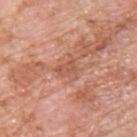– follow-up — catalogued during a skin exam; not biopsied
– location — the upper back
– illumination — white-light illumination
– TBP lesion metrics — an area of roughly 5.5 mm², a shape eccentricity near 0.4, and a shape-asymmetry score of about 0.45 (0 = symmetric); a mean CIELAB color near L≈56 a*≈25 b*≈31, a lesion–skin lightness drop of about 8, and a normalized border contrast of about 5.5; a detector confidence of about 100 out of 100 that the crop contains a lesion
– image — ~15 mm crop, total-body skin-cancer survey
– size — about 3 mm
– patient — male, aged around 60